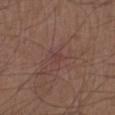Context:
Captured under white-light illumination. A male patient, aged 48–52. The lesion's longest dimension is about 1.5 mm. On the left upper arm. This image is a 15 mm lesion crop taken from a total-body photograph. Automated image analysis of the tile measured a lesion area of about 1.5 mm² and a symmetry-axis asymmetry near 0.4. It also reported an average lesion color of about L≈39 a*≈20 b*≈21 (CIELAB), about 4 CIELAB-L* units darker than the surrounding skin, and a normalized border contrast of about 4. The analysis additionally found an automated nevus-likeness rating near 0 out of 100 and a lesion-detection confidence of about 80/100.
Conclusion:
Histopathological examination showed a malignancy: nodular basal cell carcinoma.